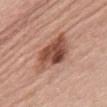anatomic site: the abdomen; acquisition: 15 mm crop, total-body photography; subject: female, aged approximately 60; lighting: white-light illumination.